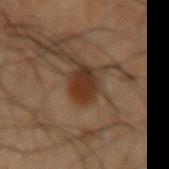  biopsy_status: not biopsied; imaged during a skin examination
  patient:
    sex: male
    age_approx: 50
  site: left upper arm
  image:
    source: total-body photography crop
    field_of_view_mm: 15
  lesion_size:
    long_diameter_mm_approx: 3.5
  lighting: cross-polarized
  automated_metrics:
    area_mm2_approx: 7.5
    eccentricity: 0.65
    cielab_L: 24
    cielab_a: 15
    cielab_b: 23
    vs_skin_darker_L: 9.0
    vs_skin_contrast_norm: 10.0